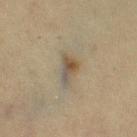biopsy status: no biopsy performed (imaged during a skin exam)
location: the left thigh
automated lesion analysis: an outline eccentricity of about 0.85 (0 = round, 1 = elongated); a mean CIELAB color near L≈42 a*≈8 b*≈23 and a normalized border contrast of about 7
subject: female, aged approximately 55
diameter: ≈3.5 mm
image: total-body-photography crop, ~15 mm field of view